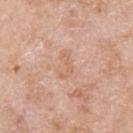Clinical impression:
Recorded during total-body skin imaging; not selected for excision or biopsy.